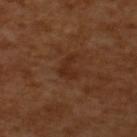Image and clinical context:
Approximately 3 mm at its widest. The patient is a male aged 63–67. A 15 mm close-up extracted from a 3D total-body photography capture. Automated tile analysis of the lesion measured an automated nevus-likeness rating near 5 out of 100 and lesion-presence confidence of about 100/100. Imaged with cross-polarized lighting.